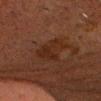Findings:
• follow-up — no biopsy performed (imaged during a skin exam)
• anatomic site — the head or neck
• lighting — cross-polarized illumination
• image source — ~15 mm crop, total-body skin-cancer survey
• subject — male, aged around 75